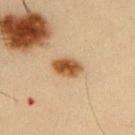Part of a total-body skin-imaging series; this lesion was reviewed on a skin check and was not flagged for biopsy. About 3.5 mm across. A 15 mm close-up extracted from a 3D total-body photography capture. Captured under cross-polarized illumination. The lesion is on the upper back. Automated tile analysis of the lesion measured a mean CIELAB color near L≈48 a*≈19 b*≈35 and a normalized border contrast of about 11. The software also gave a border-irregularity index near 1/10, a within-lesion color-variation index near 5/10, and peripheral color asymmetry of about 1.5. The software also gave a nevus-likeness score of about 100/100 and a detector confidence of about 100 out of 100 that the crop contains a lesion. The subject is a male roughly 35 years of age.